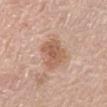A roughly 15 mm field-of-view crop from a total-body skin photograph.
Captured under white-light illumination.
The lesion is located on the abdomen.
The recorded lesion diameter is about 4 mm.
An algorithmic analysis of the crop reported an area of roughly 10 mm², a shape eccentricity near 0.75, and a shape-asymmetry score of about 0.25 (0 = symmetric). The analysis additionally found a border-irregularity index near 3/10 and a color-variation rating of about 3/10.
The patient is a male about 80 years old.